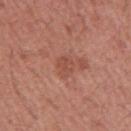This lesion was catalogued during total-body skin photography and was not selected for biopsy.
Approximately 3 mm at its widest.
Located on the left upper arm.
The tile uses white-light illumination.
Cropped from a whole-body photographic skin survey; the tile spans about 15 mm.
The patient is a male aged 43–47.
Automated tile analysis of the lesion measured a footprint of about 4.5 mm², an eccentricity of roughly 0.7, and two-axis asymmetry of about 0.25.